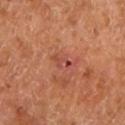follow-up — imaged on a skin check; not biopsied
site — the left lower leg
automated lesion analysis — a footprint of about 2.5 mm² and a symmetry-axis asymmetry near 0.55; a mean CIELAB color near L≈46 a*≈29 b*≈29, a lesion–skin lightness drop of about 8, and a lesion-to-skin contrast of about 6 (normalized; higher = more distinct); a border-irregularity rating of about 6/10 and internal color variation of about 0 on a 0–10 scale
lesion diameter — about 2.5 mm
acquisition — ~15 mm crop, total-body skin-cancer survey
subject — approximately 65 years of age
illumination — cross-polarized illumination